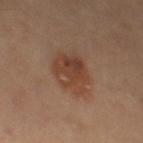* follow-up — catalogued during a skin exam; not biopsied
* imaging modality — total-body-photography crop, ~15 mm field of view
* location — the left thigh
* TBP lesion metrics — a footprint of about 14 mm², an outline eccentricity of about 0.6 (0 = round, 1 = elongated), and two-axis asymmetry of about 0.2
* patient — male, approximately 60 years of age
* diameter — ≈5 mm